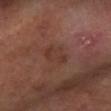<case>
  <biopsy_status>not biopsied; imaged during a skin examination</biopsy_status>
  <lighting>cross-polarized</lighting>
  <patient>
    <sex>male</sex>
    <age_approx>55</age_approx>
  </patient>
  <automated_metrics>
    <border_irregularity_0_10>3.0</border_irregularity_0_10>
    <color_variation_0_10>2.5</color_variation_0_10>
    <peripheral_color_asymmetry>0.5</peripheral_color_asymmetry>
    <nevus_likeness_0_100>0</nevus_likeness_0_100>
  </automated_metrics>
  <lesion_size>
    <long_diameter_mm_approx>3.5</long_diameter_mm_approx>
  </lesion_size>
  <image>
    <source>total-body photography crop</source>
    <field_of_view_mm>15</field_of_view_mm>
  </image>
  <site>left forearm</site>
</case>